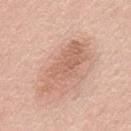The tile uses white-light illumination.
A 15 mm crop from a total-body photograph taken for skin-cancer surveillance.
A male subject in their mid- to late 50s.
Longest diameter approximately 7.5 mm.
The lesion is on the mid back.